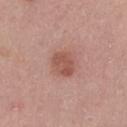The lesion was photographed on a routine skin check and not biopsied; there is no pathology result. The tile uses white-light illumination. From the left thigh. A 15 mm close-up tile from a total-body photography series done for melanoma screening. A female patient aged approximately 50. About 3.5 mm across.